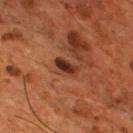workup = no biopsy performed (imaged during a skin exam); anatomic site = the chest; patient = male, aged approximately 55; image source = ~15 mm tile from a whole-body skin photo.